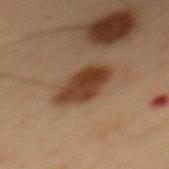Q: Was a biopsy performed?
A: catalogued during a skin exam; not biopsied
Q: Lesion size?
A: about 5.5 mm
Q: Illumination type?
A: cross-polarized illumination
Q: What are the patient's age and sex?
A: male, about 55 years old
Q: What is the anatomic site?
A: the mid back
Q: What is the imaging modality?
A: ~15 mm crop, total-body skin-cancer survey